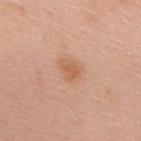Captured during whole-body skin photography for melanoma surveillance; the lesion was not biopsied.
The lesion is located on the upper back.
A male patient in their mid-50s.
This image is a 15 mm lesion crop taken from a total-body photograph.